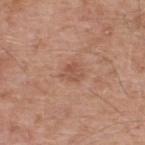Q: Was this lesion biopsied?
A: total-body-photography surveillance lesion; no biopsy
Q: Lesion location?
A: the upper back
Q: What is the imaging modality?
A: total-body-photography crop, ~15 mm field of view
Q: Who is the patient?
A: male, in their mid- to late 50s
Q: Illumination type?
A: white-light illumination
Q: Automated lesion metrics?
A: a footprint of about 4.5 mm²; border irregularity of about 3 on a 0–10 scale, a within-lesion color-variation index near 2.5/10, and a peripheral color-asymmetry measure near 1; an automated nevus-likeness rating near 0 out of 100
Q: What is the lesion's diameter?
A: about 2.5 mm